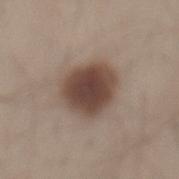Imaged during a routine full-body skin examination; the lesion was not biopsied and no histopathology is available. A male patient aged approximately 30. Located on the back. Imaged with white-light lighting. Cropped from a whole-body photographic skin survey; the tile spans about 15 mm. Measured at roughly 5 mm in maximum diameter. Automated tile analysis of the lesion measured an automated nevus-likeness rating near 100 out of 100.